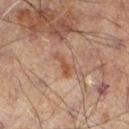No biopsy was performed on this lesion — it was imaged during a full skin examination and was not determined to be concerning. A male subject approximately 60 years of age. This is a cross-polarized tile. A 15 mm crop from a total-body photograph taken for skin-cancer surveillance. The lesion is located on the left lower leg.